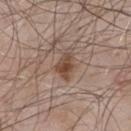workup: total-body-photography surveillance lesion; no biopsy
imaging modality: total-body-photography crop, ~15 mm field of view
site: the front of the torso
patient: male, approximately 55 years of age